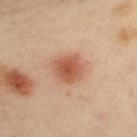Assessment:
The lesion was tiled from a total-body skin photograph and was not biopsied.
Context:
From the left upper arm. This is a cross-polarized tile. The subject is a female in their 40s. Approximately 3.5 mm at its widest. A 15 mm close-up tile from a total-body photography series done for melanoma screening.